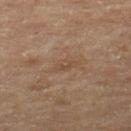No biopsy was performed on this lesion — it was imaged during a full skin examination and was not determined to be concerning. The subject is a female about 55 years old. Longest diameter approximately 2.5 mm. The lesion is located on the leg. Automated image analysis of the tile measured an area of roughly 3 mm² and two-axis asymmetry of about 0.35. It also reported an automated nevus-likeness rating near 0 out of 100 and a lesion-detection confidence of about 90/100. A region of skin cropped from a whole-body photographic capture, roughly 15 mm wide. Imaged with cross-polarized lighting.